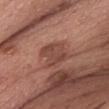The lesion was photographed on a routine skin check and not biopsied; there is no pathology result. The lesion is on the chest. Approximately 4 mm at its widest. A roughly 15 mm field-of-view crop from a total-body skin photograph. A female subject about 60 years old. This is a white-light tile.